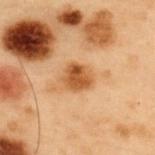Impression:
Imaged during a routine full-body skin examination; the lesion was not biopsied and no histopathology is available.
Acquisition and patient details:
Imaged with cross-polarized lighting. On the back. Longest diameter approximately 3.5 mm. A male subject, in their mid-50s. The total-body-photography lesion software estimated a mean CIELAB color near L≈45 a*≈21 b*≈36, roughly 11 lightness units darker than nearby skin, and a lesion-to-skin contrast of about 9.5 (normalized; higher = more distinct). A 15 mm crop from a total-body photograph taken for skin-cancer surveillance.